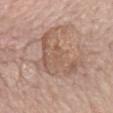The lesion was photographed on a routine skin check and not biopsied; there is no pathology result. This is a white-light tile. A female patient aged around 65. A roughly 15 mm field-of-view crop from a total-body skin photograph. The lesion is located on the back.